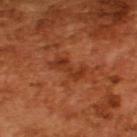Assessment:
Captured during whole-body skin photography for melanoma surveillance; the lesion was not biopsied.
Image and clinical context:
Cropped from a whole-body photographic skin survey; the tile spans about 15 mm. A male patient, aged 63–67. Automated image analysis of the tile measured a lesion–skin lightness drop of about 8 and a normalized border contrast of about 6.5. It also reported a nevus-likeness score of about 0/100 and a detector confidence of about 100 out of 100 that the crop contains a lesion.